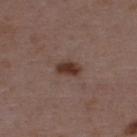automated lesion analysis: an eccentricity of roughly 0.8 and a symmetry-axis asymmetry near 0.25; roughly 12 lightness units darker than nearby skin and a normalized border contrast of about 11
imaging modality: ~15 mm crop, total-body skin-cancer survey
tile lighting: white-light
body site: the upper back
patient: female, approximately 45 years of age